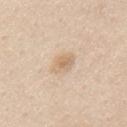acquisition: ~15 mm crop, total-body skin-cancer survey | lighting: white-light | automated lesion analysis: a shape eccentricity near 0.65; a mean CIELAB color near L≈69 a*≈15 b*≈32, a lesion–skin lightness drop of about 8, and a normalized border contrast of about 5.5 | location: the mid back | patient: female, aged 43–47 | size: about 2.5 mm.